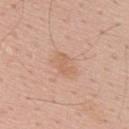location=the upper back
lesion diameter=about 3 mm
patient=male, aged around 55
imaging modality=~15 mm crop, total-body skin-cancer survey
image-analysis metrics=an area of roughly 4.5 mm² and a symmetry-axis asymmetry near 0.3; a within-lesion color-variation index near 1/10 and peripheral color asymmetry of about 0.5